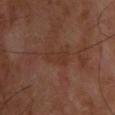This lesion was catalogued during total-body skin photography and was not selected for biopsy.
The lesion is on the back.
A male patient, aged 53–57.
The lesion-visualizer software estimated a lesion color around L≈30 a*≈19 b*≈24 in CIELAB and roughly 5 lightness units darker than nearby skin. The analysis additionally found a classifier nevus-likeness of about 0/100 and lesion-presence confidence of about 100/100.
Longest diameter approximately 3.5 mm.
A region of skin cropped from a whole-body photographic capture, roughly 15 mm wide.
The tile uses cross-polarized illumination.